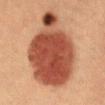No biopsy was performed on this lesion — it was imaged during a full skin examination and was not determined to be concerning. Captured under cross-polarized illumination. A female subject, aged approximately 40. From the front of the torso. This image is a 15 mm lesion crop taken from a total-body photograph. The lesion's longest dimension is about 11.5 mm.